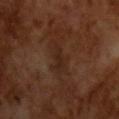A male subject, about 65 years old. Longest diameter approximately 3 mm. Cropped from a total-body skin-imaging series; the visible field is about 15 mm. The lesion-visualizer software estimated a classifier nevus-likeness of about 0/100.